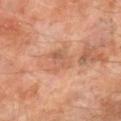Impression: The lesion was tiled from a total-body skin photograph and was not biopsied. Acquisition and patient details: Approximately 3.5 mm at its widest. This is a cross-polarized tile. On the leg. A roughly 15 mm field-of-view crop from a total-body skin photograph. The patient is a male aged approximately 60.